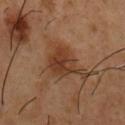Context:
A male subject, approximately 55 years of age. The total-body-photography lesion software estimated a footprint of about 11 mm², an eccentricity of roughly 0.45, and a shape-asymmetry score of about 0.3 (0 = symmetric). The analysis additionally found an average lesion color of about L≈37 a*≈21 b*≈30 (CIELAB) and a normalized border contrast of about 8. The analysis additionally found a color-variation rating of about 4/10. The software also gave a nevus-likeness score of about 95/100 and lesion-presence confidence of about 100/100. About 4.5 mm across. The tile uses cross-polarized illumination. A region of skin cropped from a whole-body photographic capture, roughly 15 mm wide. From the chest.